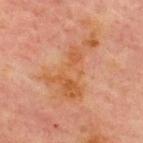biopsy status: no biopsy performed (imaged during a skin exam) | image: ~15 mm tile from a whole-body skin photo | subject: male, approximately 70 years of age | location: the chest | size: ~8 mm (longest diameter) | tile lighting: cross-polarized.